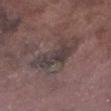{"image": {"source": "total-body photography crop", "field_of_view_mm": 15}, "patient": {"sex": "male", "age_approx": 75}, "site": "left lower leg"}